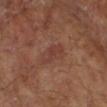Q: Was a biopsy performed?
A: total-body-photography surveillance lesion; no biopsy
Q: How was this image acquired?
A: 15 mm crop, total-body photography
Q: Lesion location?
A: the left forearm
Q: Who is the patient?
A: male, aged approximately 65